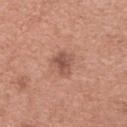{"biopsy_status": "not biopsied; imaged during a skin examination", "site": "upper back", "image": {"source": "total-body photography crop", "field_of_view_mm": 15}, "lighting": "white-light", "patient": {"sex": "female", "age_approx": 60}, "lesion_size": {"long_diameter_mm_approx": 3.0}}